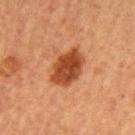Recorded during total-body skin imaging; not selected for excision or biopsy.
From the left upper arm.
Approximately 5 mm at its widest.
A female subject aged around 55.
An algorithmic analysis of the crop reported an area of roughly 13 mm², a shape eccentricity near 0.75, and a symmetry-axis asymmetry near 0.2. The analysis additionally found a nevus-likeness score of about 100/100 and a lesion-detection confidence of about 100/100.
Captured under cross-polarized illumination.
A 15 mm close-up extracted from a 3D total-body photography capture.